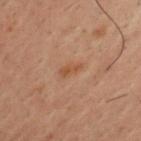Clinical impression:
Captured during whole-body skin photography for melanoma surveillance; the lesion was not biopsied.
Acquisition and patient details:
The tile uses cross-polarized illumination. A male subject in their 30s. A region of skin cropped from a whole-body photographic capture, roughly 15 mm wide. From the chest.